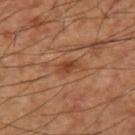Captured during whole-body skin photography for melanoma surveillance; the lesion was not biopsied. The lesion is on the right thigh. The recorded lesion diameter is about 2.5 mm. The subject is a male aged 58 to 62. The total-body-photography lesion software estimated a shape eccentricity near 0.85 and a symmetry-axis asymmetry near 0.2. It also reported internal color variation of about 1.5 on a 0–10 scale. This image is a 15 mm lesion crop taken from a total-body photograph. The tile uses cross-polarized illumination.